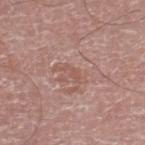biopsy_status: not biopsied; imaged during a skin examination
site: leg
image:
  source: total-body photography crop
  field_of_view_mm: 15
lesion_size:
  long_diameter_mm_approx: 2.5
automated_metrics:
  area_mm2_approx: 2.0
  eccentricity: 0.9
patient:
  sex: male
  age_approx: 75